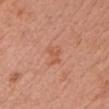| feature | finding |
|---|---|
| biopsy status | total-body-photography surveillance lesion; no biopsy |
| patient | female, aged around 65 |
| anatomic site | the chest |
| image source | ~15 mm crop, total-body skin-cancer survey |